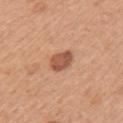Located on the left upper arm. A roughly 15 mm field-of-view crop from a total-body skin photograph. A female subject, roughly 35 years of age. The tile uses white-light illumination. The total-body-photography lesion software estimated a lesion-detection confidence of about 100/100.